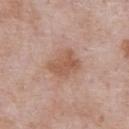Cropped from a whole-body photographic skin survey; the tile spans about 15 mm. The lesion-visualizer software estimated a symmetry-axis asymmetry near 0.25. The analysis additionally found internal color variation of about 2.5 on a 0–10 scale and peripheral color asymmetry of about 1. From the front of the torso. About 4 mm across. A male subject, approximately 55 years of age. Imaged with white-light lighting.